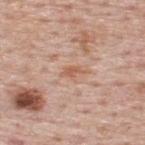Approximately 3 mm at its widest. Located on the upper back. Captured under white-light illumination. The patient is a male aged 73 to 77. An algorithmic analysis of the crop reported an automated nevus-likeness rating near 0 out of 100. A region of skin cropped from a whole-body photographic capture, roughly 15 mm wide.